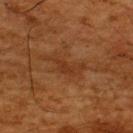Recorded during total-body skin imaging; not selected for excision or biopsy. Longest diameter approximately 4.5 mm. The tile uses cross-polarized illumination. A 15 mm close-up extracted from a 3D total-body photography capture. The subject is a male aged approximately 65. Located on the upper back.